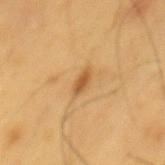<record>
  <biopsy_status>not biopsied; imaged during a skin examination</biopsy_status>
  <automated_metrics>
    <cielab_L>55</cielab_L>
    <cielab_a>21</cielab_a>
    <cielab_b>43</cielab_b>
    <vs_skin_darker_L>12.0</vs_skin_darker_L>
    <vs_skin_contrast_norm>8.0</vs_skin_contrast_norm>
    <border_irregularity_0_10>3.0</border_irregularity_0_10>
    <peripheral_color_asymmetry>0.0</peripheral_color_asymmetry>
    <nevus_likeness_0_100>55</nevus_likeness_0_100>
    <lesion_detection_confidence_0_100>100</lesion_detection_confidence_0_100>
  </automated_metrics>
  <site>mid back</site>
  <image>
    <source>total-body photography crop</source>
    <field_of_view_mm>15</field_of_view_mm>
  </image>
  <lighting>cross-polarized</lighting>
  <lesion_size>
    <long_diameter_mm_approx>3.0</long_diameter_mm_approx>
  </lesion_size>
  <patient>
    <sex>male</sex>
    <age_approx>60</age_approx>
  </patient>
</record>